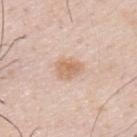Findings:
- biopsy status: imaged on a skin check; not biopsied
- size: ≈3.5 mm
- location: the upper back
- image: 15 mm crop, total-body photography
- patient: male, about 40 years old